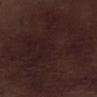Clinical impression:
The lesion was photographed on a routine skin check and not biopsied; there is no pathology result.
Clinical summary:
Cropped from a total-body skin-imaging series; the visible field is about 15 mm. The lesion is located on the right lower leg. The patient is a male aged 68 to 72.